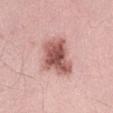Recorded during total-body skin imaging; not selected for excision or biopsy. The lesion is located on the abdomen. A 15 mm close-up extracted from a 3D total-body photography capture. Measured at roughly 5.5 mm in maximum diameter. Automated tile analysis of the lesion measured two-axis asymmetry of about 0.3. It also reported about 16 CIELAB-L* units darker than the surrounding skin and a normalized lesion–skin contrast near 10. A male patient about 30 years old.